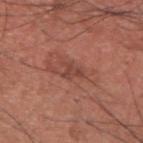No biopsy was performed on this lesion — it was imaged during a full skin examination and was not determined to be concerning. This image is a 15 mm lesion crop taken from a total-body photograph. From the upper back. The lesion-visualizer software estimated a border-irregularity rating of about 6.5/10, internal color variation of about 0.5 on a 0–10 scale, and radial color variation of about 0. The analysis additionally found a detector confidence of about 85 out of 100 that the crop contains a lesion. A male patient about 35 years old. Longest diameter approximately 3 mm.